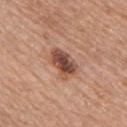notes: no biopsy performed (imaged during a skin exam) | acquisition: ~15 mm tile from a whole-body skin photo | subject: male, aged around 60 | location: the chest | TBP lesion metrics: a footprint of about 8.5 mm², a shape eccentricity near 0.8, and two-axis asymmetry of about 0.25; a lesion color around L≈49 a*≈22 b*≈28 in CIELAB and a lesion-to-skin contrast of about 10 (normalized; higher = more distinct) | lesion size: about 4 mm.